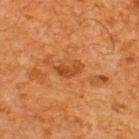Q: Was a biopsy performed?
A: no biopsy performed (imaged during a skin exam)
Q: How was this image acquired?
A: total-body-photography crop, ~15 mm field of view
Q: Lesion size?
A: about 3 mm
Q: Illumination type?
A: cross-polarized
Q: Patient demographics?
A: male, in their mid-60s
Q: Lesion location?
A: the upper back
Q: What did automated image analysis measure?
A: a shape eccentricity near 0.85 and a symmetry-axis asymmetry near 0.5; a mean CIELAB color near L≈39 a*≈25 b*≈37, roughly 8 lightness units darker than nearby skin, and a normalized lesion–skin contrast near 7; an automated nevus-likeness rating near 0 out of 100 and a detector confidence of about 100 out of 100 that the crop contains a lesion